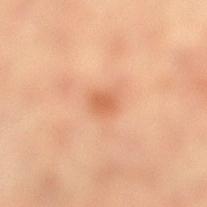{
  "biopsy_status": "not biopsied; imaged during a skin examination",
  "lighting": "cross-polarized",
  "lesion_size": {
    "long_diameter_mm_approx": 2.5
  },
  "patient": {
    "sex": "female",
    "age_approx": 65
  },
  "site": "left lower leg",
  "automated_metrics": {
    "border_irregularity_0_10": 2.5,
    "color_variation_0_10": 1.5,
    "peripheral_color_asymmetry": 0.5
  },
  "image": {
    "source": "total-body photography crop",
    "field_of_view_mm": 15
  }
}